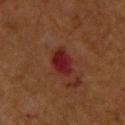| field | value |
|---|---|
| follow-up | imaged on a skin check; not biopsied |
| subject | male, aged approximately 55 |
| body site | the upper back |
| imaging modality | 15 mm crop, total-body photography |